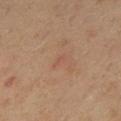follow-up=no biopsy performed (imaged during a skin exam) | subject=male, aged around 60 | imaging modality=~15 mm tile from a whole-body skin photo | site=the mid back.